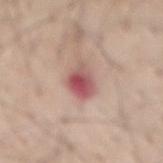The lesion was photographed on a routine skin check and not biopsied; there is no pathology result. The tile uses white-light illumination. Automated image analysis of the tile measured a shape eccentricity near 0.75 and a symmetry-axis asymmetry near 0.25. And it measured a mean CIELAB color near L≈55 a*≈26 b*≈22 and a normalized lesion–skin contrast near 9.5. The recorded lesion diameter is about 4 mm. The lesion is located on the mid back. Cropped from a whole-body photographic skin survey; the tile spans about 15 mm. A male subject, roughly 55 years of age.